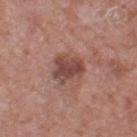Imaged during a routine full-body skin examination; the lesion was not biopsied and no histopathology is available. The total-body-photography lesion software estimated a footprint of about 9.5 mm² and a shape-asymmetry score of about 0.3 (0 = symmetric). It also reported a border-irregularity index near 3/10, a within-lesion color-variation index near 4/10, and peripheral color asymmetry of about 1.5. The analysis additionally found a detector confidence of about 100 out of 100 that the crop contains a lesion. A male patient aged 58–62. Cropped from a whole-body photographic skin survey; the tile spans about 15 mm. The tile uses white-light illumination. The recorded lesion diameter is about 4 mm. On the right thigh.